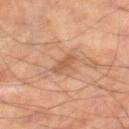Background:
Located on the right lower leg. The tile uses cross-polarized illumination. An algorithmic analysis of the crop reported a classifier nevus-likeness of about 0/100. Approximately 2.5 mm at its widest. The subject is a male aged 53 to 57. A 15 mm close-up tile from a total-body photography series done for melanoma screening.